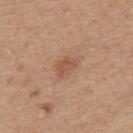| field | value |
|---|---|
| location | the upper back |
| lesion size | ≈3 mm |
| lighting | white-light illumination |
| subject | female, roughly 45 years of age |
| acquisition | total-body-photography crop, ~15 mm field of view |